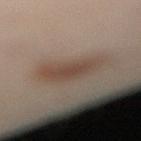Case summary:
– biopsy status: imaged on a skin check; not biopsied
– image: ~15 mm crop, total-body skin-cancer survey
– subject: female, aged approximately 30
– body site: the leg
– tile lighting: cross-polarized
– automated metrics: an outline eccentricity of about 0.65 (0 = round, 1 = elongated) and a shape-asymmetry score of about 0.25 (0 = symmetric); a border-irregularity rating of about 3/10, a color-variation rating of about 3/10, and a peripheral color-asymmetry measure near 0.5; a nevus-likeness score of about 80/100
– diameter: ≈5.5 mm